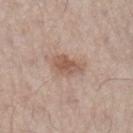Captured during whole-body skin photography for melanoma surveillance; the lesion was not biopsied. A roughly 15 mm field-of-view crop from a total-body skin photograph. Captured under white-light illumination. On the right lower leg. The lesion-visualizer software estimated a footprint of about 6.5 mm², a shape eccentricity near 0.75, and a symmetry-axis asymmetry near 0.35. And it measured an average lesion color of about L≈56 a*≈19 b*≈27 (CIELAB) and a lesion–skin lightness drop of about 10. The software also gave a within-lesion color-variation index near 3/10 and radial color variation of about 1. It also reported an automated nevus-likeness rating near 40 out of 100 and lesion-presence confidence of about 100/100. The patient is a male about 35 years old. About 3.5 mm across.